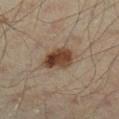An algorithmic analysis of the crop reported border irregularity of about 2.5 on a 0–10 scale and radial color variation of about 2. The patient is a male approximately 45 years of age. The tile uses cross-polarized illumination. The lesion is on the left lower leg. A lesion tile, about 15 mm wide, cut from a 3D total-body photograph.